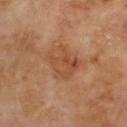Imaged during a routine full-body skin examination; the lesion was not biopsied and no histopathology is available. From the upper back. Cropped from a total-body skin-imaging series; the visible field is about 15 mm. The patient is a male roughly 70 years of age. The lesion's longest dimension is about 5 mm. Automated tile analysis of the lesion measured a shape eccentricity near 0.7 and a symmetry-axis asymmetry near 0.4. The software also gave a border-irregularity index near 5/10.